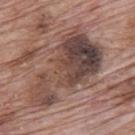Assessment:
The lesion was photographed on a routine skin check and not biopsied; there is no pathology result.
Background:
Located on the mid back. A male patient aged approximately 70. A region of skin cropped from a whole-body photographic capture, roughly 15 mm wide.